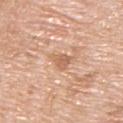Notes:
* imaging modality — total-body-photography crop, ~15 mm field of view
* illumination — white-light
* TBP lesion metrics — a border-irregularity rating of about 3/10, a color-variation rating of about 2/10, and peripheral color asymmetry of about 1; lesion-presence confidence of about 100/100
* subject — male, aged 63 to 67
* location — the upper back